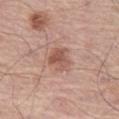Part of a total-body skin-imaging series; this lesion was reviewed on a skin check and was not flagged for biopsy. The lesion is located on the left thigh. The subject is a male aged 73–77. Captured under white-light illumination. A roughly 15 mm field-of-view crop from a total-body skin photograph. Longest diameter approximately 3 mm.